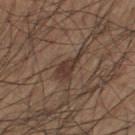Clinical impression: Part of a total-body skin-imaging series; this lesion was reviewed on a skin check and was not flagged for biopsy. Background: Imaged with white-light lighting. Cropped from a whole-body photographic skin survey; the tile spans about 15 mm. The lesion is located on the left thigh. Approximately 3.5 mm at its widest. A male subject aged 53 to 57. Automated tile analysis of the lesion measured a footprint of about 5 mm², a shape eccentricity near 0.85, and a shape-asymmetry score of about 0.45 (0 = symmetric). The analysis additionally found a border-irregularity index near 5/10, internal color variation of about 3 on a 0–10 scale, and a peripheral color-asymmetry measure near 1.